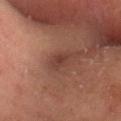Impression:
Part of a total-body skin-imaging series; this lesion was reviewed on a skin check and was not flagged for biopsy.
Background:
On the head or neck. The tile uses cross-polarized illumination. The recorded lesion diameter is about 2.5 mm. A 15 mm close-up extracted from a 3D total-body photography capture. A male patient, in their 60s.